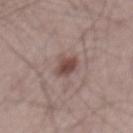Assessment:
Imaged during a routine full-body skin examination; the lesion was not biopsied and no histopathology is available.
Acquisition and patient details:
Approximately 3 mm at its widest. A lesion tile, about 15 mm wide, cut from a 3D total-body photograph. On the mid back. Imaged with white-light lighting. A male patient, in their mid-60s. The lesion-visualizer software estimated a footprint of about 5 mm², a shape eccentricity near 0.8, and two-axis asymmetry of about 0.2. And it measured a within-lesion color-variation index near 3/10 and radial color variation of about 1. It also reported a nevus-likeness score of about 90/100 and a lesion-detection confidence of about 100/100.